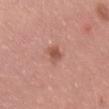Impression:
The lesion was photographed on a routine skin check and not biopsied; there is no pathology result.
Image and clinical context:
Located on the lower back. A male patient, aged around 40. A close-up tile cropped from a whole-body skin photograph, about 15 mm across.